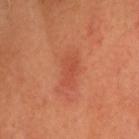Impression:
Part of a total-body skin-imaging series; this lesion was reviewed on a skin check and was not flagged for biopsy.
Context:
A 15 mm close-up extracted from a 3D total-body photography capture. A male subject roughly 65 years of age. From the head or neck. Captured under cross-polarized illumination. About 4 mm across. Automated image analysis of the tile measured a lesion area of about 6.5 mm² and a shape eccentricity near 0.85.